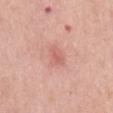<tbp_lesion>
<biopsy_status>not biopsied; imaged during a skin examination</biopsy_status>
<site>mid back</site>
<patient>
  <sex>male</sex>
  <age_approx>75</age_approx>
</patient>
<automated_metrics>
  <area_mm2_approx>4.5</area_mm2_approx>
  <eccentricity>0.8</eccentricity>
  <shape_asymmetry>0.25</shape_asymmetry>
</automated_metrics>
<image>
  <source>total-body photography crop</source>
  <field_of_view_mm>15</field_of_view_mm>
</image>
<lighting>white-light</lighting>
</tbp_lesion>